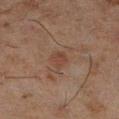<lesion>
  <biopsy_status>not biopsied; imaged during a skin examination</biopsy_status>
  <automated_metrics>
    <area_mm2_approx>3.5</area_mm2_approx>
    <eccentricity>0.7</eccentricity>
    <shape_asymmetry>0.25</shape_asymmetry>
    <cielab_L>33</cielab_L>
    <cielab_a>17</cielab_a>
    <cielab_b>23</cielab_b>
    <vs_skin_darker_L>5.0</vs_skin_darker_L>
    <vs_skin_contrast_norm>5.5</vs_skin_contrast_norm>
    <nevus_likeness_0_100>15</nevus_likeness_0_100>
    <lesion_detection_confidence_0_100>100</lesion_detection_confidence_0_100>
  </automated_metrics>
  <lesion_size>
    <long_diameter_mm_approx>2.5</long_diameter_mm_approx>
  </lesion_size>
  <patient>
    <sex>male</sex>
    <age_approx>45</age_approx>
  </patient>
  <site>right lower leg</site>
  <image>
    <source>total-body photography crop</source>
    <field_of_view_mm>15</field_of_view_mm>
  </image>
</lesion>